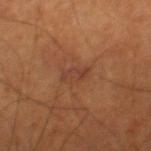* workup: total-body-photography surveillance lesion; no biopsy
* lesion diameter: about 3 mm
* imaging modality: total-body-photography crop, ~15 mm field of view
* anatomic site: the right thigh
* TBP lesion metrics: an area of roughly 4.5 mm² and a symmetry-axis asymmetry near 0.3; border irregularity of about 3 on a 0–10 scale, a color-variation rating of about 3/10, and a peripheral color-asymmetry measure near 1
* subject: male, in their 70s
* lighting: cross-polarized illumination